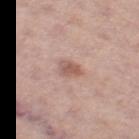{"lighting": "white-light", "site": "left thigh", "image": {"source": "total-body photography crop", "field_of_view_mm": 15}, "automated_metrics": {"eccentricity": 0.8, "shape_asymmetry": 0.25, "cielab_L": 57, "cielab_a": 21, "cielab_b": 25, "vs_skin_darker_L": 10.0, "vs_skin_contrast_norm": 7.0, "nevus_likeness_0_100": 25, "lesion_detection_confidence_0_100": 100}, "patient": {"sex": "female", "age_approx": 60}}